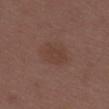{"biopsy_status": "not biopsied; imaged during a skin examination", "automated_metrics": {"area_mm2_approx": 9.5, "eccentricity": 0.65, "shape_asymmetry": 0.15, "cielab_L": 40, "cielab_a": 18, "cielab_b": 24, "vs_skin_contrast_norm": 5.0, "nevus_likeness_0_100": 10, "lesion_detection_confidence_0_100": 100}, "image": {"source": "total-body photography crop", "field_of_view_mm": 15}, "lighting": "white-light", "patient": {"sex": "female", "age_approx": 30}, "site": "upper back"}